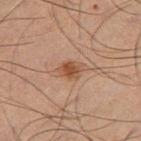Background: A male patient, in their mid-60s. A roughly 15 mm field-of-view crop from a total-body skin photograph. The lesion is on the right thigh.